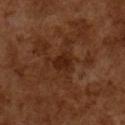The lesion was photographed on a routine skin check and not biopsied; there is no pathology result. The recorded lesion diameter is about 3.5 mm. A 15 mm close-up extracted from a 3D total-body photography capture. Captured under cross-polarized illumination. A male patient, aged around 65. The lesion-visualizer software estimated a footprint of about 6 mm² and two-axis asymmetry of about 0.45. The software also gave an automated nevus-likeness rating near 0 out of 100.